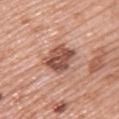{"biopsy_status": "not biopsied; imaged during a skin examination", "lighting": "white-light", "image": {"source": "total-body photography crop", "field_of_view_mm": 15}, "patient": {"sex": "female", "age_approx": 60}, "lesion_size": {"long_diameter_mm_approx": 5.0}, "site": "upper back"}